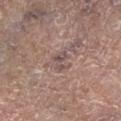Notes:
* notes — total-body-photography surveillance lesion; no biopsy
* illumination — white-light illumination
* anatomic site — the right lower leg
* subject — male, about 70 years old
* imaging modality — total-body-photography crop, ~15 mm field of view
* lesion size — ~2.5 mm (longest diameter)